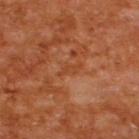Assessment:
This lesion was catalogued during total-body skin photography and was not selected for biopsy.
Context:
A male patient aged 63–67. On the upper back. A close-up tile cropped from a whole-body skin photograph, about 15 mm across. Imaged with cross-polarized lighting. Automated image analysis of the tile measured a classifier nevus-likeness of about 0/100 and a detector confidence of about 100 out of 100 that the crop contains a lesion.